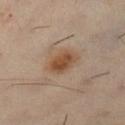Notes:
- biopsy status: catalogued during a skin exam; not biopsied
- subject: female, aged 43–47
- tile lighting: cross-polarized
- image: 15 mm crop, total-body photography
- diameter: about 4 mm
- location: the left lower leg
- automated metrics: an area of roughly 8.5 mm², an eccentricity of roughly 0.7, and a shape-asymmetry score of about 0.15 (0 = symmetric); a lesion color around L≈50 a*≈18 b*≈31 in CIELAB, about 10 CIELAB-L* units darker than the surrounding skin, and a lesion-to-skin contrast of about 8.5 (normalized; higher = more distinct); a border-irregularity index near 1.5/10, a color-variation rating of about 4.5/10, and radial color variation of about 1.5; an automated nevus-likeness rating near 95 out of 100 and a lesion-detection confidence of about 100/100